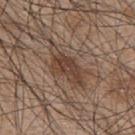notes=imaged on a skin check; not biopsied | lesion size=≈6.5 mm | image=~15 mm tile from a whole-body skin photo | location=the upper back | subject=male, aged around 45 | tile lighting=white-light illumination | automated metrics=an average lesion color of about L≈42 a*≈16 b*≈26 (CIELAB), about 9 CIELAB-L* units darker than the surrounding skin, and a normalized lesion–skin contrast near 7.5.